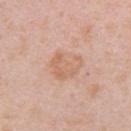This lesion was catalogued during total-body skin photography and was not selected for biopsy.
The lesion's longest dimension is about 4 mm.
A region of skin cropped from a whole-body photographic capture, roughly 15 mm wide.
Imaged with white-light lighting.
A female patient in their mid- to late 40s.
Automated tile analysis of the lesion measured a shape eccentricity near 0.55 and two-axis asymmetry of about 0.2. It also reported a lesion color around L≈64 a*≈20 b*≈31 in CIELAB, a lesion–skin lightness drop of about 7, and a lesion-to-skin contrast of about 5 (normalized; higher = more distinct).
From the arm.